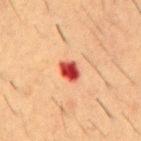<tbp_lesion>
  <biopsy_status>not biopsied; imaged during a skin examination</biopsy_status>
  <lesion_size>
    <long_diameter_mm_approx>3.0</long_diameter_mm_approx>
  </lesion_size>
  <lighting>cross-polarized</lighting>
  <automated_metrics>
    <cielab_L>46</cielab_L>
    <cielab_a>37</cielab_a>
    <cielab_b>33</cielab_b>
    <vs_skin_contrast_norm>13.0</vs_skin_contrast_norm>
    <color_variation_0_10>5.5</color_variation_0_10>
    <peripheral_color_asymmetry>1.5</peripheral_color_asymmetry>
    <nevus_likeness_0_100>0</nevus_likeness_0_100>
  </automated_metrics>
  <image>
    <source>total-body photography crop</source>
    <field_of_view_mm>15</field_of_view_mm>
  </image>
  <site>chest</site>
  <patient>
    <sex>male</sex>
    <age_approx>55</age_approx>
  </patient>
</tbp_lesion>